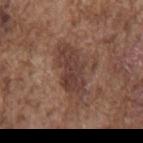biopsy status = imaged on a skin check; not biopsied
size = about 6.5 mm
automated metrics = about 9 CIELAB-L* units darker than the surrounding skin; border irregularity of about 4.5 on a 0–10 scale and a within-lesion color-variation index near 3/10
acquisition = 15 mm crop, total-body photography
subject = male, aged 73–77
site = the back